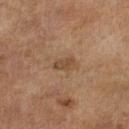The lesion was tiled from a total-body skin photograph and was not biopsied.
An algorithmic analysis of the crop reported a lesion color around L≈40 a*≈16 b*≈29 in CIELAB and about 7 CIELAB-L* units darker than the surrounding skin. The analysis additionally found a within-lesion color-variation index near 0.5/10. It also reported a classifier nevus-likeness of about 20/100 and a lesion-detection confidence of about 100/100.
The lesion is on the left lower leg.
A region of skin cropped from a whole-body photographic capture, roughly 15 mm wide.
The patient is a female aged approximately 60.
About 2.5 mm across.
This is a cross-polarized tile.